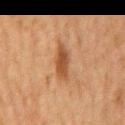anatomic site — the mid back
imaging modality — total-body-photography crop, ~15 mm field of view
subject — male, in their mid- to late 70s